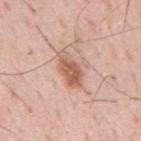Notes:
- workup — total-body-photography surveillance lesion; no biopsy
- diameter — ~4.5 mm (longest diameter)
- imaging modality — ~15 mm tile from a whole-body skin photo
- anatomic site — the mid back
- patient — male, aged around 55